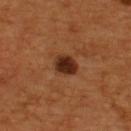Case summary:
* biopsy status — no biopsy performed (imaged during a skin exam)
* site — the upper back
* TBP lesion metrics — a lesion area of about 6 mm², an eccentricity of roughly 0.55, and a symmetry-axis asymmetry near 0.15; a lesion color around L≈27 a*≈21 b*≈28 in CIELAB and a lesion–skin lightness drop of about 13; a border-irregularity rating of about 1.5/10, a color-variation rating of about 3/10, and peripheral color asymmetry of about 1; a classifier nevus-likeness of about 80/100 and lesion-presence confidence of about 100/100
* patient — male, in their mid-50s
* image — ~15 mm crop, total-body skin-cancer survey
* lesion size — ≈3 mm